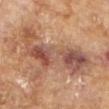A female patient, approximately 80 years of age.
Imaged with cross-polarized lighting.
About 9.5 mm across.
A 15 mm close-up tile from a total-body photography series done for melanoma screening.
From the right forearm.